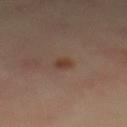The lesion was tiled from a total-body skin photograph and was not biopsied. The lesion's longest dimension is about 2 mm. Imaged with cross-polarized lighting. On the mid back. A female subject, aged approximately 40. A lesion tile, about 15 mm wide, cut from a 3D total-body photograph.